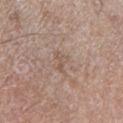Impression:
Part of a total-body skin-imaging series; this lesion was reviewed on a skin check and was not flagged for biopsy.
Image and clinical context:
Cropped from a whole-body photographic skin survey; the tile spans about 15 mm. Located on the left lower leg. Automated tile analysis of the lesion measured an area of roughly 2.5 mm², a shape eccentricity near 0.75, and a symmetry-axis asymmetry near 0.6. And it measured an average lesion color of about L≈54 a*≈17 b*≈26 (CIELAB), about 6 CIELAB-L* units darker than the surrounding skin, and a normalized border contrast of about 5. And it measured a classifier nevus-likeness of about 0/100 and lesion-presence confidence of about 100/100. The recorded lesion diameter is about 2.5 mm. The patient is a male in their mid- to late 50s.